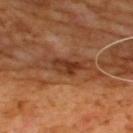{"biopsy_status": "not biopsied; imaged during a skin examination", "patient": {"sex": "male", "age_approx": 65}, "image": {"source": "total-body photography crop", "field_of_view_mm": 15}, "site": "upper back", "lighting": "cross-polarized", "lesion_size": {"long_diameter_mm_approx": 3.5}}